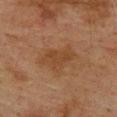biopsy status = imaged on a skin check; not biopsied | image = total-body-photography crop, ~15 mm field of view | subject = male, aged approximately 75 | site = the upper back | tile lighting = cross-polarized illumination | size = ~5.5 mm (longest diameter).